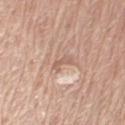Findings:
- follow-up · imaged on a skin check; not biopsied
- image · total-body-photography crop, ~15 mm field of view
- automated metrics · a mean CIELAB color near L≈60 a*≈19 b*≈28 and a lesion-to-skin contrast of about 5.5 (normalized; higher = more distinct); a border-irregularity index near 5/10, internal color variation of about 0 on a 0–10 scale, and radial color variation of about 0
- lighting · white-light
- patient · male, aged 78–82
- location · the left upper arm